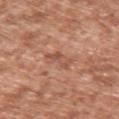Q: Is there a histopathology result?
A: no biopsy performed (imaged during a skin exam)
Q: Automated lesion metrics?
A: an area of roughly 5 mm², an outline eccentricity of about 0.95 (0 = round, 1 = elongated), and a shape-asymmetry score of about 0.3 (0 = symmetric); a border-irregularity rating of about 4/10, internal color variation of about 3.5 on a 0–10 scale, and radial color variation of about 1; a nevus-likeness score of about 5/100 and a detector confidence of about 100 out of 100 that the crop contains a lesion
Q: What is the lesion's diameter?
A: ≈4 mm
Q: Where on the body is the lesion?
A: the right upper arm
Q: What kind of image is this?
A: total-body-photography crop, ~15 mm field of view
Q: Who is the patient?
A: male, roughly 45 years of age